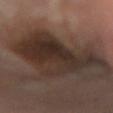Part of a total-body skin-imaging series; this lesion was reviewed on a skin check and was not flagged for biopsy. The lesion is on the chest. The total-body-photography lesion software estimated a lesion area of about 42 mm². The analysis additionally found an average lesion color of about L≈25 a*≈12 b*≈18 (CIELAB) and roughly 9 lightness units darker than nearby skin. The software also gave border irregularity of about 6 on a 0–10 scale and internal color variation of about 5 on a 0–10 scale. The analysis additionally found a classifier nevus-likeness of about 5/100 and a detector confidence of about 85 out of 100 that the crop contains a lesion. A region of skin cropped from a whole-body photographic capture, roughly 15 mm wide. A female patient aged around 60. About 9 mm across.